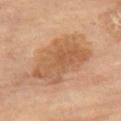Q: Was this lesion biopsied?
A: no biopsy performed (imaged during a skin exam)
Q: Who is the patient?
A: female, aged 68 to 72
Q: How large is the lesion?
A: about 8 mm
Q: What is the anatomic site?
A: the right thigh
Q: What is the imaging modality?
A: ~15 mm tile from a whole-body skin photo
Q: What did automated image analysis measure?
A: a border-irregularity rating of about 3.5/10 and a within-lesion color-variation index near 4/10
Q: Illumination type?
A: cross-polarized illumination